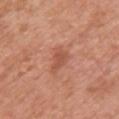Recorded during total-body skin imaging; not selected for excision or biopsy. The lesion's longest dimension is about 3.5 mm. This is a white-light tile. The patient is a female aged around 50. This image is a 15 mm lesion crop taken from a total-body photograph. From the chest.